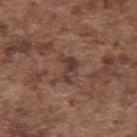Assessment:
Imaged during a routine full-body skin examination; the lesion was not biopsied and no histopathology is available.
Context:
The lesion is located on the upper back. A male patient, approximately 75 years of age. The lesion-visualizer software estimated a color-variation rating of about 3.5/10. A region of skin cropped from a whole-body photographic capture, roughly 15 mm wide.